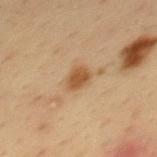notes: total-body-photography surveillance lesion; no biopsy
patient: male, aged 33–37
size: ≈2.5 mm
anatomic site: the mid back
tile lighting: cross-polarized illumination
image: 15 mm crop, total-body photography